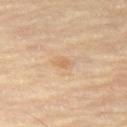Q: Was this lesion biopsied?
A: imaged on a skin check; not biopsied
Q: What is the imaging modality?
A: 15 mm crop, total-body photography
Q: Who is the patient?
A: female, about 75 years old
Q: Lesion location?
A: the leg